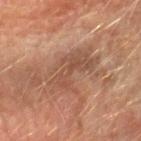workup: no biopsy performed (imaged during a skin exam)
imaging modality: ~15 mm tile from a whole-body skin photo
subject: male, in their mid- to late 60s
anatomic site: the left forearm
lighting: cross-polarized